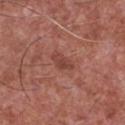Part of a total-body skin-imaging series; this lesion was reviewed on a skin check and was not flagged for biopsy.